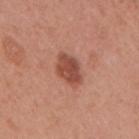Assessment: Captured during whole-body skin photography for melanoma surveillance; the lesion was not biopsied. Context: The patient is a female aged 38–42. A 15 mm crop from a total-body photograph taken for skin-cancer surveillance. On the upper back. The lesion's longest dimension is about 4 mm. Automated image analysis of the tile measured a lesion-detection confidence of about 100/100. This is a white-light tile.